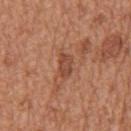Clinical impression: This lesion was catalogued during total-body skin photography and was not selected for biopsy. Image and clinical context: From the mid back. Automated tile analysis of the lesion measured a lesion color around L≈47 a*≈25 b*≈31 in CIELAB and about 9 CIELAB-L* units darker than the surrounding skin. It also reported border irregularity of about 2 on a 0–10 scale, a within-lesion color-variation index near 2/10, and a peripheral color-asymmetry measure near 0.5. A male subject roughly 65 years of age. A 15 mm close-up tile from a total-body photography series done for melanoma screening.